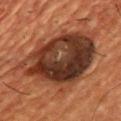Imaged during a routine full-body skin examination; the lesion was not biopsied and no histopathology is available.
This is a cross-polarized tile.
Located on the upper back.
A 15 mm close-up extracted from a 3D total-body photography capture.
A male patient aged 53–57.
The lesion-visualizer software estimated a footprint of about 50 mm² and an outline eccentricity of about 0.7 (0 = round, 1 = elongated). The software also gave an automated nevus-likeness rating near 5 out of 100 and a lesion-detection confidence of about 95/100.
The lesion's longest dimension is about 10.5 mm.